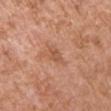{"biopsy_status": "not biopsied; imaged during a skin examination", "patient": {"sex": "male", "age_approx": 75}, "site": "left upper arm", "image": {"source": "total-body photography crop", "field_of_view_mm": 15}}